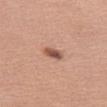Part of a total-body skin-imaging series; this lesion was reviewed on a skin check and was not flagged for biopsy.
The patient is a female in their mid- to late 50s.
The lesion is on the leg.
An algorithmic analysis of the crop reported a border-irregularity rating of about 2/10 and internal color variation of about 5.5 on a 0–10 scale.
A close-up tile cropped from a whole-body skin photograph, about 15 mm across.
Imaged with white-light lighting.